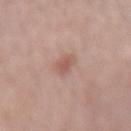No biopsy was performed on this lesion — it was imaged during a full skin examination and was not determined to be concerning.
From the right forearm.
The lesion's longest dimension is about 2.5 mm.
The lesion-visualizer software estimated a lesion-detection confidence of about 100/100.
A female subject aged approximately 40.
A roughly 15 mm field-of-view crop from a total-body skin photograph.
Imaged with white-light lighting.